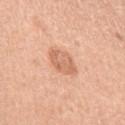* image source — ~15 mm tile from a whole-body skin photo
* patient — female, aged approximately 35
* lesion size — about 3.5 mm
* site — the left upper arm